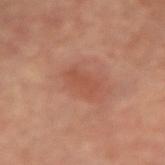biopsy status = no biopsy performed (imaged during a skin exam)
TBP lesion metrics = radial color variation of about 0.5; an automated nevus-likeness rating near 10 out of 100
lesion diameter = ~3.5 mm (longest diameter)
image = ~15 mm tile from a whole-body skin photo
illumination = cross-polarized illumination
patient = female, roughly 65 years of age
body site = the left arm